Cropped from a whole-body photographic skin survey; the tile spans about 15 mm · the patient is aged around 55 · the lesion is on the chest · captured under cross-polarized illumination: 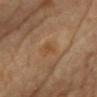| feature | finding |
|---|---|
| pathology | an atypical melanocytic neoplasm — a lesion of indeterminate malignant potential |